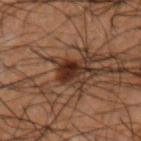| key | value |
|---|---|
| workup | imaged on a skin check; not biopsied |
| size | about 3.5 mm |
| lighting | cross-polarized illumination |
| acquisition | ~15 mm tile from a whole-body skin photo |
| patient | male, aged around 50 |
| site | the right forearm |